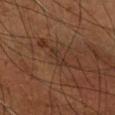Q: Is there a histopathology result?
A: total-body-photography surveillance lesion; no biopsy
Q: Automated lesion metrics?
A: a within-lesion color-variation index near 0/10 and peripheral color asymmetry of about 0; an automated nevus-likeness rating near 0 out of 100 and a detector confidence of about 65 out of 100 that the crop contains a lesion
Q: Lesion location?
A: the right forearm
Q: How was this image acquired?
A: 15 mm crop, total-body photography
Q: Patient demographics?
A: male, aged 58 to 62